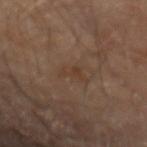Impression: Captured during whole-body skin photography for melanoma surveillance; the lesion was not biopsied.